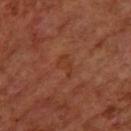| key | value |
|---|---|
| follow-up | catalogued during a skin exam; not biopsied |
| size | ≈3 mm |
| patient | female, aged approximately 65 |
| tile lighting | cross-polarized illumination |
| anatomic site | the left forearm |
| image | ~15 mm tile from a whole-body skin photo |